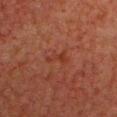| key | value |
|---|---|
| notes | no biopsy performed (imaged during a skin exam) |
| body site | the head or neck |
| lighting | cross-polarized |
| subject | male, aged 58–62 |
| size | ≈3 mm |
| image source | ~15 mm tile from a whole-body skin photo |